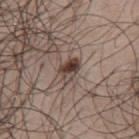Assessment:
No biopsy was performed on this lesion — it was imaged during a full skin examination and was not determined to be concerning.
Clinical summary:
A region of skin cropped from a whole-body photographic capture, roughly 15 mm wide. The lesion is on the back. The subject is a male aged 48–52.